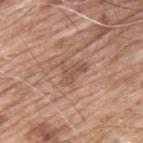<tbp_lesion>
<biopsy_status>not biopsied; imaged during a skin examination</biopsy_status>
<lesion_size>
  <long_diameter_mm_approx>3.5</long_diameter_mm_approx>
</lesion_size>
<site>back</site>
<image>
  <source>total-body photography crop</source>
  <field_of_view_mm>15</field_of_view_mm>
</image>
<patient>
  <sex>male</sex>
  <age_approx>60</age_approx>
</patient>
<automated_metrics>
  <area_mm2_approx>5.5</area_mm2_approx>
  <eccentricity>0.65</eccentricity>
  <shape_asymmetry>0.55</shape_asymmetry>
  <color_variation_0_10>2.0</color_variation_0_10>
  <peripheral_color_asymmetry>0.5</peripheral_color_asymmetry>
  <nevus_likeness_0_100>0</nevus_likeness_0_100>
  <lesion_detection_confidence_0_100>100</lesion_detection_confidence_0_100>
</automated_metrics>
</tbp_lesion>